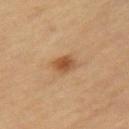Findings:
– biopsy status · total-body-photography surveillance lesion; no biopsy
– body site · the leg
– size · about 2.5 mm
– patient · female, in their 70s
– image source · total-body-photography crop, ~15 mm field of view
– lighting · cross-polarized illumination